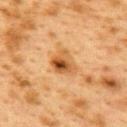Q: Was a biopsy performed?
A: catalogued during a skin exam; not biopsied